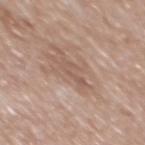| field | value |
|---|---|
| workup | catalogued during a skin exam; not biopsied |
| image source | total-body-photography crop, ~15 mm field of view |
| TBP lesion metrics | a lesion area of about 4.5 mm², an eccentricity of roughly 0.9, and a symmetry-axis asymmetry near 0.45; a detector confidence of about 75 out of 100 that the crop contains a lesion |
| size | about 3.5 mm |
| site | the mid back |
| subject | male, about 60 years old |
| lighting | white-light |